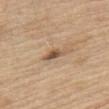Assessment: Captured during whole-body skin photography for melanoma surveillance; the lesion was not biopsied. Acquisition and patient details: On the front of the torso. Imaged with white-light lighting. A lesion tile, about 15 mm wide, cut from a 3D total-body photograph. A male subject, about 70 years old.